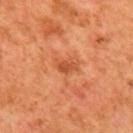The lesion was photographed on a routine skin check and not biopsied; there is no pathology result. Automated image analysis of the tile measured a footprint of about 3.5 mm² and two-axis asymmetry of about 0.35. It also reported an average lesion color of about L≈51 a*≈32 b*≈41 (CIELAB) and a normalized border contrast of about 6.5. It also reported a border-irregularity index near 3/10, internal color variation of about 1.5 on a 0–10 scale, and peripheral color asymmetry of about 0.5. And it measured an automated nevus-likeness rating near 0 out of 100 and a lesion-detection confidence of about 100/100. A 15 mm crop from a total-body photograph taken for skin-cancer surveillance. The lesion is on the back. The patient is a male aged around 65. Imaged with cross-polarized lighting.